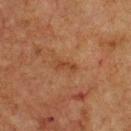| feature | finding |
|---|---|
| workup | catalogued during a skin exam; not biopsied |
| illumination | cross-polarized illumination |
| location | the front of the torso |
| lesion diameter | ≈3 mm |
| TBP lesion metrics | a border-irregularity index near 4/10 and a color-variation rating of about 0/10; a nevus-likeness score of about 0/100 |
| patient | male, in their mid-70s |
| acquisition | ~15 mm tile from a whole-body skin photo |